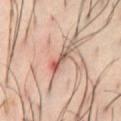Context: Located on the front of the torso. This is a cross-polarized tile. Measured at roughly 4 mm in maximum diameter. A roughly 15 mm field-of-view crop from a total-body skin photograph. A male patient aged 38–42.